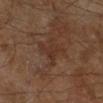Q: Is there a histopathology result?
A: imaged on a skin check; not biopsied
Q: Lesion location?
A: the leg
Q: What are the patient's age and sex?
A: male, aged 58–62
Q: What kind of image is this?
A: ~15 mm tile from a whole-body skin photo
Q: Lesion size?
A: about 4.5 mm